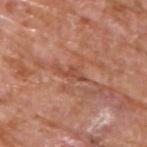No biopsy was performed on this lesion — it was imaged during a full skin examination and was not determined to be concerning. A male patient roughly 65 years of age. A 15 mm close-up extracted from a 3D total-body photography capture. Located on the upper back.